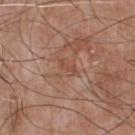Impression:
The lesion was tiled from a total-body skin photograph and was not biopsied.
Clinical summary:
This is a white-light tile. The lesion is on the chest. Automated image analysis of the tile measured a footprint of about 2 mm² and a symmetry-axis asymmetry near 0.45. The analysis additionally found a lesion color around L≈49 a*≈22 b*≈30 in CIELAB and a lesion–skin lightness drop of about 6. It also reported a border-irregularity rating of about 5/10, a color-variation rating of about 0/10, and a peripheral color-asymmetry measure near 0. The software also gave a nevus-likeness score of about 0/100. A male patient in their mid- to late 60s. This image is a 15 mm lesion crop taken from a total-body photograph. Measured at roughly 2.5 mm in maximum diameter.